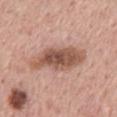Part of a total-body skin-imaging series; this lesion was reviewed on a skin check and was not flagged for biopsy. On the mid back. Captured under white-light illumination. This image is a 15 mm lesion crop taken from a total-body photograph. The patient is a male aged around 60. The recorded lesion diameter is about 8 mm.